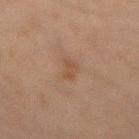Impression:
Captured during whole-body skin photography for melanoma surveillance; the lesion was not biopsied.
Background:
Located on the mid back. The subject is a male approximately 45 years of age. Imaged with cross-polarized lighting. This image is a 15 mm lesion crop taken from a total-body photograph.